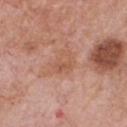Imaged during a routine full-body skin examination; the lesion was not biopsied and no histopathology is available. A 15 mm close-up extracted from a 3D total-body photography capture. A male subject aged 58 to 62. The lesion is on the chest. Imaged with white-light lighting. Longest diameter approximately 3.5 mm. An algorithmic analysis of the crop reported a footprint of about 7 mm² and an eccentricity of roughly 0.7. It also reported an average lesion color of about L≈56 a*≈23 b*≈32 (CIELAB), roughly 6 lightness units darker than nearby skin, and a normalized lesion–skin contrast near 5. The software also gave internal color variation of about 3.5 on a 0–10 scale and radial color variation of about 1.5.